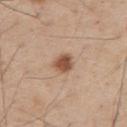Image and clinical context:
A lesion tile, about 15 mm wide, cut from a 3D total-body photograph. The lesion is located on the upper back. This is a white-light tile. A male subject, roughly 55 years of age.